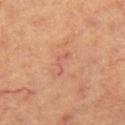Clinical impression: No biopsy was performed on this lesion — it was imaged during a full skin examination and was not determined to be concerning. Context: Approximately 3 mm at its widest. Located on the leg. This is a cross-polarized tile. A female patient approximately 60 years of age. A region of skin cropped from a whole-body photographic capture, roughly 15 mm wide.